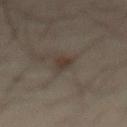The total-body-photography lesion software estimated a classifier nevus-likeness of about 30/100.
Imaged with cross-polarized lighting.
A male subject about 50 years old.
A 15 mm close-up extracted from a 3D total-body photography capture.
The lesion is on the front of the torso.